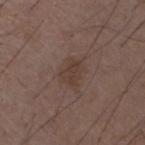• workup — catalogued during a skin exam; not biopsied
• patient — male, aged around 50
• lesion size — ~3 mm (longest diameter)
• image — total-body-photography crop, ~15 mm field of view
• TBP lesion metrics — a mean CIELAB color near L≈38 a*≈15 b*≈22 and about 6 CIELAB-L* units darker than the surrounding skin; border irregularity of about 2.5 on a 0–10 scale, a color-variation rating of about 1.5/10, and peripheral color asymmetry of about 0.5; lesion-presence confidence of about 100/100
• anatomic site — the right upper arm
• lighting — white-light illumination